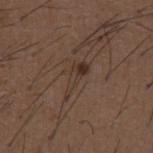notes: imaged on a skin check; not biopsied
subject: male, roughly 50 years of age
image source: total-body-photography crop, ~15 mm field of view
illumination: white-light
lesion size: ~4.5 mm (longest diameter)
body site: the abdomen